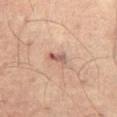subject: male, approximately 60 years of age
automated metrics: a lesion color around L≈54 a*≈19 b*≈25 in CIELAB, a lesion–skin lightness drop of about 10, and a normalized lesion–skin contrast near 7; an automated nevus-likeness rating near 0 out of 100 and a detector confidence of about 95 out of 100 that the crop contains a lesion
diameter: ~3 mm (longest diameter)
body site: the front of the torso
acquisition: ~15 mm crop, total-body skin-cancer survey